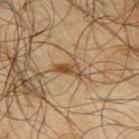No biopsy was performed on this lesion — it was imaged during a full skin examination and was not determined to be concerning. Located on the upper back. The patient is a male about 65 years old. A 15 mm close-up extracted from a 3D total-body photography capture.